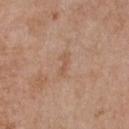Q: Was a biopsy performed?
A: total-body-photography surveillance lesion; no biopsy
Q: How was this image acquired?
A: 15 mm crop, total-body photography
Q: Where on the body is the lesion?
A: the chest
Q: What are the patient's age and sex?
A: female, about 40 years old